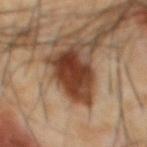No biopsy was performed on this lesion — it was imaged during a full skin examination and was not determined to be concerning. Imaged with cross-polarized lighting. Measured at roughly 6.5 mm in maximum diameter. On the abdomen. A male patient, approximately 65 years of age. A 15 mm close-up extracted from a 3D total-body photography capture. The total-body-photography lesion software estimated a footprint of about 19 mm², an outline eccentricity of about 0.75 (0 = round, 1 = elongated), and two-axis asymmetry of about 0.25. The software also gave about 17 CIELAB-L* units darker than the surrounding skin and a lesion-to-skin contrast of about 13.5 (normalized; higher = more distinct). And it measured a border-irregularity index near 3.5/10, internal color variation of about 5 on a 0–10 scale, and radial color variation of about 1.5. It also reported an automated nevus-likeness rating near 95 out of 100 and a lesion-detection confidence of about 100/100.